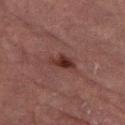notes: catalogued during a skin exam; not biopsied
subject: female, aged around 70
anatomic site: the left thigh
TBP lesion metrics: an area of roughly 5 mm² and a symmetry-axis asymmetry near 0.3; a mean CIELAB color near L≈27 a*≈19 b*≈20, about 8 CIELAB-L* units darker than the surrounding skin, and a normalized lesion–skin contrast near 9; an automated nevus-likeness rating near 85 out of 100
illumination: cross-polarized illumination
acquisition: 15 mm crop, total-body photography